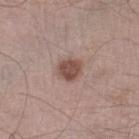No biopsy was performed on this lesion — it was imaged during a full skin examination and was not determined to be concerning. This is a white-light tile. Located on the right lower leg. The total-body-photography lesion software estimated a border-irregularity rating of about 1.5/10 and a peripheral color-asymmetry measure near 1. A male patient about 55 years old. Cropped from a whole-body photographic skin survey; the tile spans about 15 mm.